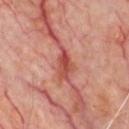Q: Was this lesion biopsied?
A: no biopsy performed (imaged during a skin exam)
Q: What is the lesion's diameter?
A: about 3 mm
Q: Who is the patient?
A: male, in their mid-60s
Q: What is the anatomic site?
A: the chest
Q: What lighting was used for the tile?
A: cross-polarized illumination
Q: What kind of image is this?
A: ~15 mm tile from a whole-body skin photo